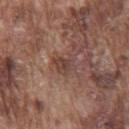Case summary:
• workup · catalogued during a skin exam; not biopsied
• automated metrics · an area of roughly 5 mm², an eccentricity of roughly 0.65, and two-axis asymmetry of about 0.3; about 8 CIELAB-L* units darker than the surrounding skin and a lesion-to-skin contrast of about 7 (normalized; higher = more distinct); a border-irregularity index near 3.5/10, a within-lesion color-variation index near 2.5/10, and a peripheral color-asymmetry measure near 1
• tile lighting · white-light
• patient · male, aged 73–77
• image · total-body-photography crop, ~15 mm field of view
• body site · the chest